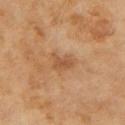| feature | finding |
|---|---|
| body site | the left upper arm |
| imaging modality | ~15 mm crop, total-body skin-cancer survey |
| subject | female, aged around 60 |